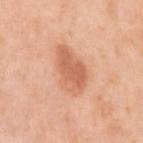Clinical impression: Part of a total-body skin-imaging series; this lesion was reviewed on a skin check and was not flagged for biopsy. Acquisition and patient details: A 15 mm close-up extracted from a 3D total-body photography capture. Imaged with white-light lighting. Automated image analysis of the tile measured an average lesion color of about L≈64 a*≈26 b*≈36 (CIELAB), a lesion–skin lightness drop of about 10, and a normalized lesion–skin contrast near 6.5. The subject is a female approximately 40 years of age. On the arm. Approximately 5.5 mm at its widest.